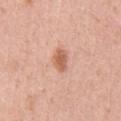– notes: imaged on a skin check; not biopsied
– illumination: white-light
– image source: ~15 mm tile from a whole-body skin photo
– patient: male, aged 53 to 57
– diameter: ≈3 mm
– anatomic site: the chest